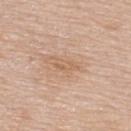  biopsy_status: not biopsied; imaged during a skin examination
  site: upper back
  patient:
    sex: male
    age_approx: 80
  lesion_size:
    long_diameter_mm_approx: 3.0
  image:
    source: total-body photography crop
    field_of_view_mm: 15
  lighting: white-light